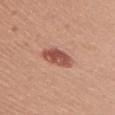No biopsy was performed on this lesion — it was imaged during a full skin examination and was not determined to be concerning.
About 4 mm across.
Captured under white-light illumination.
Automated image analysis of the tile measured a footprint of about 7.5 mm², a shape eccentricity near 0.75, and a symmetry-axis asymmetry near 0.2. The analysis additionally found a mean CIELAB color near L≈52 a*≈27 b*≈28 and a lesion–skin lightness drop of about 13. It also reported an automated nevus-likeness rating near 95 out of 100 and lesion-presence confidence of about 100/100.
A roughly 15 mm field-of-view crop from a total-body skin photograph.
A male patient roughly 55 years of age.
On the right upper arm.